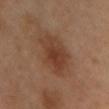Q: Is there a histopathology result?
A: catalogued during a skin exam; not biopsied